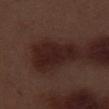workup=total-body-photography surveillance lesion; no biopsy
image=~15 mm tile from a whole-body skin photo
location=the right thigh
subject=male, aged around 70